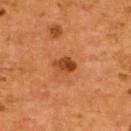Notes:
- biopsy status — no biopsy performed (imaged during a skin exam)
- patient — male, about 55 years old
- acquisition — total-body-photography crop, ~15 mm field of view
- anatomic site — the upper back